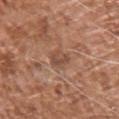{
  "biopsy_status": "not biopsied; imaged during a skin examination",
  "patient": {
    "sex": "male",
    "age_approx": 75
  },
  "lighting": "white-light",
  "site": "chest",
  "lesion_size": {
    "long_diameter_mm_approx": 3.0
  },
  "automated_metrics": {
    "area_mm2_approx": 3.0,
    "eccentricity": 0.85
  },
  "image": {
    "source": "total-body photography crop",
    "field_of_view_mm": 15
  }
}